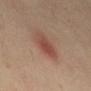<tbp_lesion>
  <biopsy_status>not biopsied; imaged during a skin examination</biopsy_status>
  <image>
    <source>total-body photography crop</source>
    <field_of_view_mm>15</field_of_view_mm>
  </image>
  <patient>
    <sex>female</sex>
    <age_approx>40</age_approx>
  </patient>
  <lesion_size>
    <long_diameter_mm_approx>4.0</long_diameter_mm_approx>
  </lesion_size>
  <lighting>cross-polarized</lighting>
  <site>abdomen</site>
</tbp_lesion>